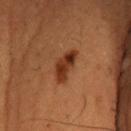Notes:
• biopsy status: total-body-photography surveillance lesion; no biopsy
• diameter: ≈5 mm
• body site: the head or neck
• patient: male, aged 48–52
• lighting: cross-polarized
• automated metrics: a border-irregularity rating of about 4/10 and internal color variation of about 4.5 on a 0–10 scale; an automated nevus-likeness rating near 85 out of 100 and lesion-presence confidence of about 100/100
• acquisition: ~15 mm tile from a whole-body skin photo